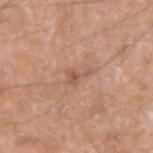Q: Was this lesion biopsied?
A: imaged on a skin check; not biopsied
Q: Illumination type?
A: white-light illumination
Q: What are the patient's age and sex?
A: male, aged approximately 55
Q: What is the anatomic site?
A: the right upper arm
Q: What is the imaging modality?
A: ~15 mm tile from a whole-body skin photo
Q: What did automated image analysis measure?
A: an area of roughly 3.5 mm², a shape eccentricity near 0.85, and a shape-asymmetry score of about 0.55 (0 = symmetric); a lesion–skin lightness drop of about 8